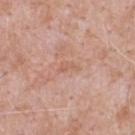Imaged during a routine full-body skin examination; the lesion was not biopsied and no histopathology is available. The patient is a male aged 48–52. A 15 mm crop from a total-body photograph taken for skin-cancer surveillance. Automated tile analysis of the lesion measured a footprint of about 2.5 mm², a shape eccentricity near 0.9, and two-axis asymmetry of about 0.4. It also reported a border-irregularity index near 4.5/10 and a peripheral color-asymmetry measure near 0. The analysis additionally found lesion-presence confidence of about 100/100. The recorded lesion diameter is about 2.5 mm. From the upper back.